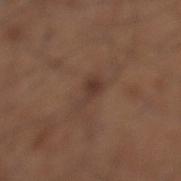Q: Lesion size?
A: ~3 mm (longest diameter)
Q: How was the tile lit?
A: white-light illumination
Q: Who is the patient?
A: male, about 60 years old
Q: What is the imaging modality?
A: total-body-photography crop, ~15 mm field of view
Q: Where on the body is the lesion?
A: the left lower leg